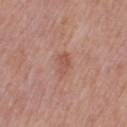Q: Is there a histopathology result?
A: imaged on a skin check; not biopsied
Q: What are the patient's age and sex?
A: female, in their 40s
Q: What is the anatomic site?
A: the right thigh
Q: What kind of image is this?
A: ~15 mm crop, total-body skin-cancer survey
Q: What did automated image analysis measure?
A: an average lesion color of about L≈53 a*≈24 b*≈27 (CIELAB), a lesion–skin lightness drop of about 8, and a lesion-to-skin contrast of about 5.5 (normalized; higher = more distinct); a classifier nevus-likeness of about 10/100
Q: What is the lesion's diameter?
A: about 2.5 mm
Q: How was the tile lit?
A: white-light illumination